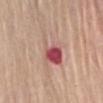* follow-up: catalogued during a skin exam; not biopsied
* lighting: white-light
* lesion diameter: about 6.5 mm
* subject: male, aged around 80
* TBP lesion metrics: a lesion area of about 13 mm², an outline eccentricity of about 0.8 (0 = round, 1 = elongated), and two-axis asymmetry of about 0.45; a mean CIELAB color near L≈56 a*≈25 b*≈24 and about 11 CIELAB-L* units darker than the surrounding skin; a border-irregularity rating of about 6.5/10, internal color variation of about 10 on a 0–10 scale, and radial color variation of about 6; an automated nevus-likeness rating near 0 out of 100 and lesion-presence confidence of about 100/100
* acquisition: 15 mm crop, total-body photography
* site: the abdomen